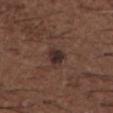follow-up: total-body-photography surveillance lesion; no biopsy
imaging modality: 15 mm crop, total-body photography
patient: male, approximately 50 years of age
body site: the back